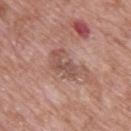Assessment:
The lesion was tiled from a total-body skin photograph and was not biopsied.
Context:
The tile uses white-light illumination. On the upper back. The total-body-photography lesion software estimated a peripheral color-asymmetry measure near 1. The software also gave lesion-presence confidence of about 100/100. The patient is a male aged 68–72. Cropped from a whole-body photographic skin survey; the tile spans about 15 mm.